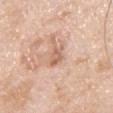<tbp_lesion>
  <biopsy_status>not biopsied; imaged during a skin examination</biopsy_status>
  <patient>
    <sex>male</sex>
    <age_approx>40</age_approx>
  </patient>
  <lesion_size>
    <long_diameter_mm_approx>2.5</long_diameter_mm_approx>
  </lesion_size>
  <site>chest</site>
  <image>
    <source>total-body photography crop</source>
    <field_of_view_mm>15</field_of_view_mm>
  </image>
  <lighting>white-light</lighting>
  <automated_metrics>
    <area_mm2_approx>4.0</area_mm2_approx>
    <eccentricity>0.75</eccentricity>
    <vs_skin_darker_L>9.0</vs_skin_darker_L>
    <vs_skin_contrast_norm>6.0</vs_skin_contrast_norm>
    <color_variation_0_10>2.0</color_variation_0_10>
    <peripheral_color_asymmetry>0.5</peripheral_color_asymmetry>
    <nevus_likeness_0_100>0</nevus_likeness_0_100>
    <lesion_detection_confidence_0_100>100</lesion_detection_confidence_0_100>
  </automated_metrics>
</tbp_lesion>